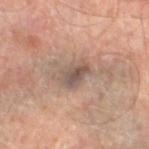Clinical impression:
No biopsy was performed on this lesion — it was imaged during a full skin examination and was not determined to be concerning.
Background:
The recorded lesion diameter is about 3.5 mm. On the left lower leg. A male subject, aged 68 to 72. A region of skin cropped from a whole-body photographic capture, roughly 15 mm wide. Captured under cross-polarized illumination. Automated tile analysis of the lesion measured an eccentricity of roughly 0.75 and two-axis asymmetry of about 0.3. The software also gave an average lesion color of about L≈50 a*≈14 b*≈22 (CIELAB), about 10 CIELAB-L* units darker than the surrounding skin, and a normalized lesion–skin contrast near 7.5. And it measured a classifier nevus-likeness of about 30/100 and lesion-presence confidence of about 95/100.